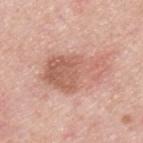Clinical impression:
No biopsy was performed on this lesion — it was imaged during a full skin examination and was not determined to be concerning.
Acquisition and patient details:
A lesion tile, about 15 mm wide, cut from a 3D total-body photograph. Located on the upper back. Measured at roughly 7.5 mm in maximum diameter. A male patient about 45 years old.